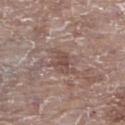Imaged during a routine full-body skin examination; the lesion was not biopsied and no histopathology is available.
A female patient, aged approximately 85 or older.
A roughly 15 mm field-of-view crop from a total-body skin photograph.
The lesion is on the left lower leg.